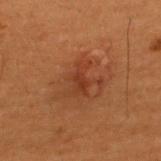The lesion was tiled from a total-body skin photograph and was not biopsied.
Cropped from a whole-body photographic skin survey; the tile spans about 15 mm.
The lesion is located on the back.
Imaged with cross-polarized lighting.
A male patient roughly 50 years of age.